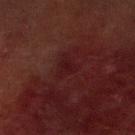Clinical impression: Imaged during a routine full-body skin examination; the lesion was not biopsied and no histopathology is available. Background: Captured under cross-polarized illumination. The recorded lesion diameter is about 3 mm. The subject is a male approximately 70 years of age. A roughly 15 mm field-of-view crop from a total-body skin photograph. The lesion is located on the right lower leg.